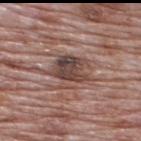The lesion was photographed on a routine skin check and not biopsied; there is no pathology result. Located on the upper back. A roughly 15 mm field-of-view crop from a total-body skin photograph. A male subject, aged around 70.